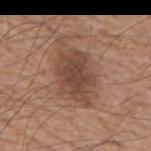The lesion was tiled from a total-body skin photograph and was not biopsied.
The lesion is located on the mid back.
The patient is a male roughly 75 years of age.
A 15 mm crop from a total-body photograph taken for skin-cancer surveillance.
This is a white-light tile.
The recorded lesion diameter is about 6 mm.